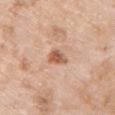The patient is a female aged around 70. The lesion-visualizer software estimated a lesion area of about 4 mm² and an outline eccentricity of about 0.75 (0 = round, 1 = elongated). The analysis additionally found an average lesion color of about L≈58 a*≈23 b*≈32 (CIELAB) and a lesion-to-skin contrast of about 8.5 (normalized; higher = more distinct). And it measured a nevus-likeness score of about 90/100 and a detector confidence of about 100 out of 100 that the crop contains a lesion. A 15 mm close-up tile from a total-body photography series done for melanoma screening. About 3 mm across. The lesion is located on the left upper arm.